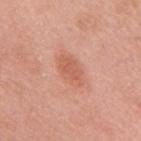subject: female, approximately 60 years of age | illumination: white-light | image source: ~15 mm tile from a whole-body skin photo | anatomic site: the back.